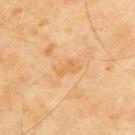Automated image analysis of the tile measured a lesion area of about 4.5 mm², an eccentricity of roughly 0.85, and two-axis asymmetry of about 0.3. The software also gave a lesion color around L≈54 a*≈17 b*≈36 in CIELAB and a normalized lesion–skin contrast near 4.5. And it measured a nevus-likeness score of about 0/100 and lesion-presence confidence of about 100/100.
A male subject aged 43–47.
The tile uses cross-polarized illumination.
A 15 mm close-up tile from a total-body photography series done for melanoma screening.
From the upper back.